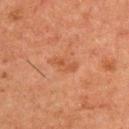Recorded during total-body skin imaging; not selected for excision or biopsy.
The tile uses cross-polarized illumination.
The patient is a male aged around 50.
A 15 mm close-up tile from a total-body photography series done for melanoma screening.
An algorithmic analysis of the crop reported an eccentricity of roughly 0.9 and two-axis asymmetry of about 0.3. The software also gave a lesion color around L≈42 a*≈22 b*≈31 in CIELAB, roughly 5 lightness units darker than nearby skin, and a lesion-to-skin contrast of about 5 (normalized; higher = more distinct). The analysis additionally found a border-irregularity index near 3.5/10 and internal color variation of about 2 on a 0–10 scale.
Located on the upper back.